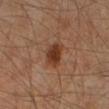Clinical impression:
Recorded during total-body skin imaging; not selected for excision or biopsy.
Context:
Captured under cross-polarized illumination. A male patient, aged approximately 60. From the left lower leg. A region of skin cropped from a whole-body photographic capture, roughly 15 mm wide.